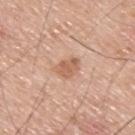Clinical impression:
Recorded during total-body skin imaging; not selected for excision or biopsy.
Acquisition and patient details:
Cropped from a whole-body photographic skin survey; the tile spans about 15 mm. A male patient roughly 70 years of age. Located on the upper back. Captured under white-light illumination. Measured at roughly 3 mm in maximum diameter. The total-body-photography lesion software estimated a footprint of about 5.5 mm², an eccentricity of roughly 0.65, and a symmetry-axis asymmetry near 0.3. The analysis additionally found a color-variation rating of about 2/10 and radial color variation of about 0.5. It also reported a nevus-likeness score of about 10/100.